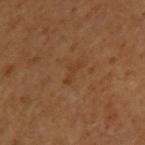Q: Is there a histopathology result?
A: total-body-photography surveillance lesion; no biopsy
Q: Who is the patient?
A: male, roughly 50 years of age
Q: How large is the lesion?
A: about 3 mm
Q: How was this image acquired?
A: 15 mm crop, total-body photography
Q: What is the anatomic site?
A: the left upper arm
Q: Automated lesion metrics?
A: an automated nevus-likeness rating near 0 out of 100 and lesion-presence confidence of about 100/100